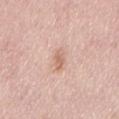Context:
Automated image analysis of the tile measured an area of roughly 3.5 mm², an eccentricity of roughly 0.9, and a symmetry-axis asymmetry near 0.2. It also reported roughly 9 lightness units darker than nearby skin and a normalized border contrast of about 6. And it measured border irregularity of about 2.5 on a 0–10 scale, internal color variation of about 1.5 on a 0–10 scale, and radial color variation of about 0.5. It also reported a classifier nevus-likeness of about 5/100 and a detector confidence of about 100 out of 100 that the crop contains a lesion. A lesion tile, about 15 mm wide, cut from a 3D total-body photograph. The lesion is on the mid back. The tile uses white-light illumination. The subject is a male aged 53–57. About 3 mm across.